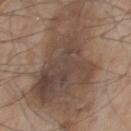Q: Was a biopsy performed?
A: imaged on a skin check; not biopsied
Q: What is the imaging modality?
A: 15 mm crop, total-body photography
Q: What lighting was used for the tile?
A: white-light
Q: What did automated image analysis measure?
A: a lesion color around L≈46 a*≈15 b*≈24 in CIELAB, roughly 11 lightness units darker than nearby skin, and a normalized border contrast of about 8
Q: What is the lesion's diameter?
A: ~12 mm (longest diameter)
Q: Patient demographics?
A: male, in their 60s
Q: What is the anatomic site?
A: the chest